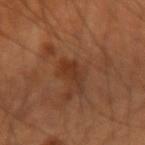Imaged during a routine full-body skin examination; the lesion was not biopsied and no histopathology is available. The lesion is located on the left arm. The lesion-visualizer software estimated a lesion color around L≈34 a*≈22 b*≈31 in CIELAB and roughly 7 lightness units darker than nearby skin. And it measured a border-irregularity rating of about 4.5/10, a color-variation rating of about 2.5/10, and radial color variation of about 0.5. The software also gave an automated nevus-likeness rating near 5 out of 100 and lesion-presence confidence of about 100/100. Measured at roughly 3.5 mm in maximum diameter. This is a cross-polarized tile. A male subject aged 48–52. A region of skin cropped from a whole-body photographic capture, roughly 15 mm wide.